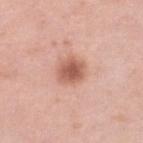lighting=white-light illumination | lesion diameter=≈3.5 mm | anatomic site=the leg | patient=female, in their 40s | automated lesion analysis=a nevus-likeness score of about 95/100 and a lesion-detection confidence of about 100/100 | imaging modality=15 mm crop, total-body photography.